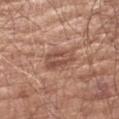This lesion was catalogued during total-body skin photography and was not selected for biopsy. Located on the left upper arm. Captured under white-light illumination. An algorithmic analysis of the crop reported an area of roughly 7.5 mm², an outline eccentricity of about 0.8 (0 = round, 1 = elongated), and a shape-asymmetry score of about 0.2 (0 = symmetric). The software also gave a mean CIELAB color near L≈50 a*≈21 b*≈28 and roughly 9 lightness units darker than nearby skin. The software also gave a border-irregularity rating of about 2/10. And it measured a lesion-detection confidence of about 95/100. A lesion tile, about 15 mm wide, cut from a 3D total-body photograph. A male patient, aged approximately 65. Approximately 4 mm at its widest.